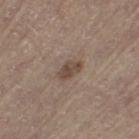  biopsy_status: not biopsied; imaged during a skin examination
  image:
    source: total-body photography crop
    field_of_view_mm: 15
  site: leg
  lesion_size:
    long_diameter_mm_approx: 3.0
  lighting: white-light
  patient:
    sex: male
    age_approx: 65
  automated_metrics:
    shape_asymmetry: 0.25
    border_irregularity_0_10: 2.0
    color_variation_0_10: 2.5
    peripheral_color_asymmetry: 1.0
    nevus_likeness_0_100: 30
    lesion_detection_confidence_0_100: 100